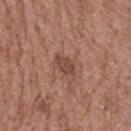notes: catalogued during a skin exam; not biopsied | image: 15 mm crop, total-body photography | size: about 3 mm | patient: male, in their 50s | TBP lesion metrics: a footprint of about 3.5 mm², an outline eccentricity of about 0.85 (0 = round, 1 = elongated), and a shape-asymmetry score of about 0.3 (0 = symmetric); a mean CIELAB color near L≈45 a*≈21 b*≈28 and roughly 9 lightness units darker than nearby skin; a classifier nevus-likeness of about 30/100 and lesion-presence confidence of about 100/100 | location: the upper back.